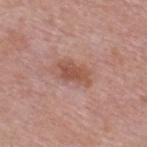Assessment:
The lesion was tiled from a total-body skin photograph and was not biopsied.
Context:
The subject is a male about 55 years old. From the back. A lesion tile, about 15 mm wide, cut from a 3D total-body photograph. Captured under white-light illumination. Approximately 4 mm at its widest.